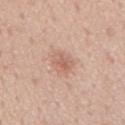Recorded during total-body skin imaging; not selected for excision or biopsy. A male patient aged 48–52. From the mid back. Cropped from a total-body skin-imaging series; the visible field is about 15 mm.